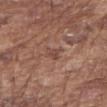Imaged during a routine full-body skin examination; the lesion was not biopsied and no histopathology is available. The patient is a male in their mid-70s. About 2.5 mm across. Captured under white-light illumination. Located on the right forearm. A lesion tile, about 15 mm wide, cut from a 3D total-body photograph.